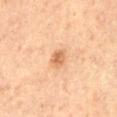follow-up = imaged on a skin check; not biopsied | location = the left thigh | lesion size = ~2.5 mm (longest diameter) | subject = male, aged 63 to 67 | image source = ~15 mm crop, total-body skin-cancer survey.